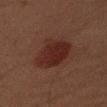notes: imaged on a skin check; not biopsied | lesion size: ≈4.5 mm | image: 15 mm crop, total-body photography | anatomic site: the right upper arm | tile lighting: cross-polarized illumination | subject: male, aged 43–47 | TBP lesion metrics: a footprint of about 15 mm² and an outline eccentricity of about 0.65 (0 = round, 1 = elongated); roughly 7 lightness units darker than nearby skin and a normalized border contrast of about 9; a border-irregularity index near 2.5/10, a color-variation rating of about 3/10, and radial color variation of about 1.